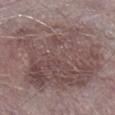follow-up=imaged on a skin check; not biopsied | lesion size=≈12 mm | location=the right lower leg | subject=male, approximately 75 years of age | acquisition=total-body-photography crop, ~15 mm field of view | TBP lesion metrics=a detector confidence of about 95 out of 100 that the crop contains a lesion.